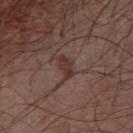<case>
  <biopsy_status>not biopsied; imaged during a skin examination</biopsy_status>
  <patient>
    <sex>male</sex>
    <age_approx>55</age_approx>
  </patient>
  <image>
    <source>total-body photography crop</source>
    <field_of_view_mm>15</field_of_view_mm>
  </image>
  <lighting>white-light</lighting>
  <site>chest</site>
</case>